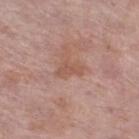biopsy status = catalogued during a skin exam; not biopsied | body site = the right thigh | image = ~15 mm tile from a whole-body skin photo | patient = female, aged 58 to 62.